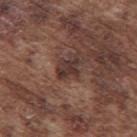Impression:
Captured during whole-body skin photography for melanoma surveillance; the lesion was not biopsied.
Image and clinical context:
The tile uses white-light illumination. The total-body-photography lesion software estimated an area of roughly 4.5 mm², an eccentricity of roughly 0.75, and a shape-asymmetry score of about 0.35 (0 = symmetric). The software also gave a border-irregularity index near 4/10, a color-variation rating of about 1/10, and peripheral color asymmetry of about 0.5. The software also gave an automated nevus-likeness rating near 5 out of 100 and a lesion-detection confidence of about 100/100. The lesion's longest dimension is about 3 mm. A close-up tile cropped from a whole-body skin photograph, about 15 mm across. Located on the upper back. A male patient about 75 years old.